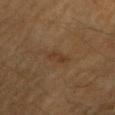This lesion was catalogued during total-body skin photography and was not selected for biopsy. A 15 mm close-up extracted from a 3D total-body photography capture. A male patient, in their mid-80s or older. The lesion is on the right upper arm. The tile uses cross-polarized illumination.